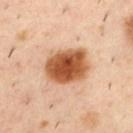Q: Was this lesion biopsied?
A: catalogued during a skin exam; not biopsied
Q: How was the tile lit?
A: cross-polarized
Q: How large is the lesion?
A: ~5.5 mm (longest diameter)
Q: What is the imaging modality?
A: 15 mm crop, total-body photography
Q: Lesion location?
A: the upper back
Q: What are the patient's age and sex?
A: male, roughly 40 years of age